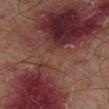Q: Is there a histopathology result?
A: catalogued during a skin exam; not biopsied
Q: How was this image acquired?
A: ~15 mm crop, total-body skin-cancer survey
Q: What is the anatomic site?
A: the left lower leg
Q: What lighting was used for the tile?
A: cross-polarized
Q: Who is the patient?
A: male, aged approximately 65